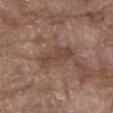image = ~15 mm crop, total-body skin-cancer survey | body site = the abdomen | patient = male, aged approximately 80.